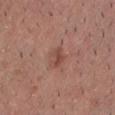<case>
  <patient>
    <sex>male</sex>
    <age_approx>25</age_approx>
  </patient>
  <lighting>white-light</lighting>
  <lesion_size>
    <long_diameter_mm_approx>2.5</long_diameter_mm_approx>
  </lesion_size>
  <image>
    <source>total-body photography crop</source>
    <field_of_view_mm>15</field_of_view_mm>
  </image>
  <site>chest</site>
</case>